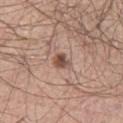Clinical impression:
Imaged during a routine full-body skin examination; the lesion was not biopsied and no histopathology is available.
Clinical summary:
A male subject aged approximately 40. From the leg. Cropped from a total-body skin-imaging series; the visible field is about 15 mm. The total-body-photography lesion software estimated a lesion area of about 3.5 mm², an outline eccentricity of about 0.75 (0 = round, 1 = elongated), and a shape-asymmetry score of about 0.25 (0 = symmetric). The software also gave a mean CIELAB color near L≈50 a*≈20 b*≈25, about 13 CIELAB-L* units darker than the surrounding skin, and a lesion-to-skin contrast of about 9 (normalized; higher = more distinct). The software also gave border irregularity of about 2 on a 0–10 scale and peripheral color asymmetry of about 1.5. The software also gave a nevus-likeness score of about 85/100 and lesion-presence confidence of about 100/100. The recorded lesion diameter is about 2.5 mm.